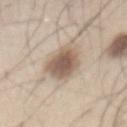site: abdomen
patient:
  sex: male
  age_approx: 45
lesion_size:
  long_diameter_mm_approx: 5.5
lighting: white-light
image:
  source: total-body photography crop
  field_of_view_mm: 15